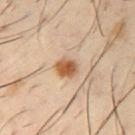This lesion was catalogued during total-body skin photography and was not selected for biopsy. The lesion-visualizer software estimated an area of roughly 5.5 mm² and an eccentricity of roughly 0.5. It also reported a normalized lesion–skin contrast near 10. The software also gave a border-irregularity index near 1/10 and peripheral color asymmetry of about 1.5. The software also gave lesion-presence confidence of about 100/100. From the right upper arm. A male subject aged around 40. Captured under cross-polarized illumination. A 15 mm close-up extracted from a 3D total-body photography capture.